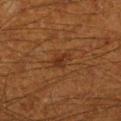<lesion>
  <biopsy_status>not biopsied; imaged during a skin examination</biopsy_status>
  <site>right lower leg</site>
  <image>
    <source>total-body photography crop</source>
    <field_of_view_mm>15</field_of_view_mm>
  </image>
  <lighting>cross-polarized</lighting>
  <lesion_size>
    <long_diameter_mm_approx>2.5</long_diameter_mm_approx>
  </lesion_size>
  <automated_metrics>
    <area_mm2_approx>4.0</area_mm2_approx>
    <eccentricity>0.7</eccentricity>
    <shape_asymmetry>0.25</shape_asymmetry>
    <cielab_L>27</cielab_L>
    <cielab_a>19</cielab_a>
    <cielab_b>28</cielab_b>
    <vs_skin_darker_L>6.0</vs_skin_darker_L>
    <vs_skin_contrast_norm>6.5</vs_skin_contrast_norm>
  </automated_metrics>
  <patient>
    <sex>male</sex>
    <age_approx>60</age_approx>
  </patient>
</lesion>